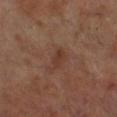Clinical impression:
The lesion was tiled from a total-body skin photograph and was not biopsied.
Image and clinical context:
The recorded lesion diameter is about 3 mm. A roughly 15 mm field-of-view crop from a total-body skin photograph. A male subject aged 68 to 72. The lesion is located on the right lower leg.